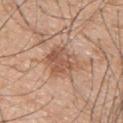Impression: This lesion was catalogued during total-body skin photography and was not selected for biopsy. Clinical summary: A male subject aged approximately 50. Located on the upper back. Automated image analysis of the tile measured a border-irregularity rating of about 3/10, a color-variation rating of about 4.5/10, and peripheral color asymmetry of about 1.5. The analysis additionally found a classifier nevus-likeness of about 20/100 and a lesion-detection confidence of about 100/100. The tile uses white-light illumination. A lesion tile, about 15 mm wide, cut from a 3D total-body photograph. Measured at roughly 4.5 mm in maximum diameter.